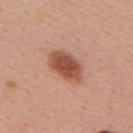Clinical impression: Recorded during total-body skin imaging; not selected for excision or biopsy. Clinical summary: Captured under white-light illumination. Automated image analysis of the tile measured a lesion color around L≈52 a*≈25 b*≈31 in CIELAB. The software also gave border irregularity of about 1.5 on a 0–10 scale, a within-lesion color-variation index near 3.5/10, and peripheral color asymmetry of about 1. The lesion is on the mid back. Cropped from a total-body skin-imaging series; the visible field is about 15 mm. A female subject, about 55 years old. The lesion's longest dimension is about 5 mm.